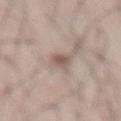{
  "biopsy_status": "not biopsied; imaged during a skin examination",
  "site": "front of the torso",
  "lighting": "white-light",
  "patient": {
    "sex": "male",
    "age_approx": 55
  },
  "automated_metrics": {
    "shape_asymmetry": 0.2,
    "cielab_L": 56,
    "cielab_a": 15,
    "cielab_b": 23,
    "vs_skin_darker_L": 11.0,
    "vs_skin_contrast_norm": 7.5,
    "border_irregularity_0_10": 2.5,
    "color_variation_0_10": 3.0,
    "nevus_likeness_0_100": 95,
    "lesion_detection_confidence_0_100": 100
  },
  "image": {
    "source": "total-body photography crop",
    "field_of_view_mm": 15
  }
}